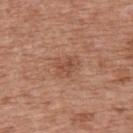A 15 mm close-up tile from a total-body photography series done for melanoma screening. Located on the upper back. This is a white-light tile. The patient is a female aged approximately 40. Measured at roughly 3 mm in maximum diameter. An algorithmic analysis of the crop reported a border-irregularity rating of about 3/10, a color-variation rating of about 2/10, and peripheral color asymmetry of about 1. It also reported a nevus-likeness score of about 5/100 and a detector confidence of about 100 out of 100 that the crop contains a lesion.